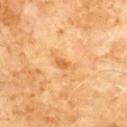{
  "biopsy_status": "not biopsied; imaged during a skin examination",
  "site": "chest",
  "image": {
    "source": "total-body photography crop",
    "field_of_view_mm": 15
  },
  "lighting": "cross-polarized",
  "patient": {
    "sex": "male",
    "age_approx": 60
  }
}